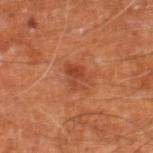Notes:
- notes: total-body-photography surveillance lesion; no biopsy
- site: the right leg
- lesion size: ≈3 mm
- acquisition: total-body-photography crop, ~15 mm field of view
- automated lesion analysis: a footprint of about 5 mm² and a shape-asymmetry score of about 0.4 (0 = symmetric)
- lighting: cross-polarized illumination
- subject: male, about 60 years old